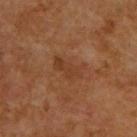This lesion was catalogued during total-body skin photography and was not selected for biopsy.
Captured under cross-polarized illumination.
A male subject, aged around 60.
A close-up tile cropped from a whole-body skin photograph, about 15 mm across.
On the back.